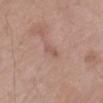biopsy status: no biopsy performed (imaged during a skin exam) | site: the arm | diameter: about 2.5 mm | imaging modality: ~15 mm crop, total-body skin-cancer survey | patient: male, about 60 years old.